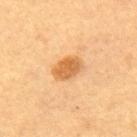Part of a total-body skin-imaging series; this lesion was reviewed on a skin check and was not flagged for biopsy. The tile uses cross-polarized illumination. A close-up tile cropped from a whole-body skin photograph, about 15 mm across. The recorded lesion diameter is about 3.5 mm. From the upper back. A female patient, aged 58 to 62.